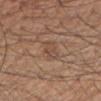{"automated_metrics": {"cielab_L": 47, "cielab_a": 18, "cielab_b": 27, "vs_skin_contrast_norm": 5.0, "nevus_likeness_0_100": 0, "lesion_detection_confidence_0_100": 65}, "lighting": "white-light", "patient": {"sex": "male", "age_approx": 55}, "site": "right upper arm", "lesion_size": {"long_diameter_mm_approx": 3.0}, "image": {"source": "total-body photography crop", "field_of_view_mm": 15}}